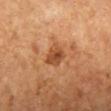<lesion>
<biopsy_status>not biopsied; imaged during a skin examination</biopsy_status>
<site>right lower leg</site>
<patient>
  <age_approx>70</age_approx>
</patient>
<image>
  <source>total-body photography crop</source>
  <field_of_view_mm>15</field_of_view_mm>
</image>
</lesion>